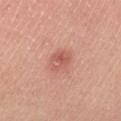Assessment:
Part of a total-body skin-imaging series; this lesion was reviewed on a skin check and was not flagged for biopsy.